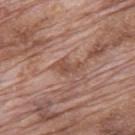Findings:
• notes · imaged on a skin check; not biopsied
• patient · male, approximately 70 years of age
• acquisition · 15 mm crop, total-body photography
• lesion size · ~3.5 mm (longest diameter)
• location · the mid back
• lighting · white-light illumination
• TBP lesion metrics · an average lesion color of about L≈50 a*≈21 b*≈27 (CIELAB); a within-lesion color-variation index near 1/10 and a peripheral color-asymmetry measure near 0.5; a classifier nevus-likeness of about 0/100